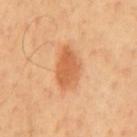{"biopsy_status": "not biopsied; imaged during a skin examination", "image": {"source": "total-body photography crop", "field_of_view_mm": 15}, "automated_metrics": {"cielab_L": 61, "cielab_a": 26, "cielab_b": 41, "vs_skin_darker_L": 11.0, "vs_skin_contrast_norm": 7.0}, "lesion_size": {"long_diameter_mm_approx": 5.0}, "patient": {"sex": "male", "age_approx": 65}, "site": "front of the torso", "lighting": "cross-polarized"}